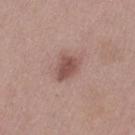follow-up = total-body-photography surveillance lesion; no biopsy | subject = female, aged 38–42 | automated metrics = a footprint of about 6.5 mm²; a mean CIELAB color near L≈51 a*≈21 b*≈23, roughly 11 lightness units darker than nearby skin, and a normalized border contrast of about 7.5; a border-irregularity rating of about 2.5/10 and radial color variation of about 1; an automated nevus-likeness rating near 70 out of 100 | illumination = white-light illumination | site = the left thigh | image source = ~15 mm tile from a whole-body skin photo.